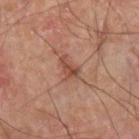Recorded during total-body skin imaging; not selected for excision or biopsy.
Located on the left forearm.
Measured at roughly 3 mm in maximum diameter.
Imaged with cross-polarized lighting.
Cropped from a whole-body photographic skin survey; the tile spans about 15 mm.
The lesion-visualizer software estimated a lesion area of about 3.5 mm² and an outline eccentricity of about 0.85 (0 = round, 1 = elongated). The analysis additionally found a lesion–skin lightness drop of about 8 and a lesion-to-skin contrast of about 7 (normalized; higher = more distinct). And it measured a border-irregularity rating of about 5/10, a within-lesion color-variation index near 3/10, and peripheral color asymmetry of about 1.5. The software also gave an automated nevus-likeness rating near 0 out of 100 and a lesion-detection confidence of about 100/100.
The patient is a male about 60 years old.